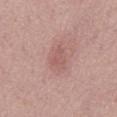Clinical summary:
A male subject in their mid-70s. The lesion-visualizer software estimated a lesion area of about 4 mm², a shape eccentricity near 0.75, and two-axis asymmetry of about 0.3. The analysis additionally found radial color variation of about 0.5. A roughly 15 mm field-of-view crop from a total-body skin photograph. Measured at roughly 2.5 mm in maximum diameter. The tile uses white-light illumination. On the abdomen.